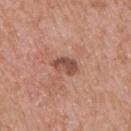biopsy status: imaged on a skin check; not biopsied | location: the back | subject: male, roughly 60 years of age | imaging modality: ~15 mm crop, total-body skin-cancer survey.